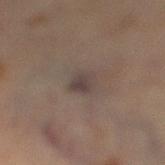Recorded during total-body skin imaging; not selected for excision or biopsy. Approximately 3 mm at its widest. A roughly 15 mm field-of-view crop from a total-body skin photograph. The lesion is on the right lower leg. A female patient roughly 70 years of age. Imaged with cross-polarized lighting. Automated image analysis of the tile measured a lesion area of about 5 mm², a shape eccentricity near 0.7, and a shape-asymmetry score of about 0.25 (0 = symmetric). The analysis additionally found a color-variation rating of about 3.5/10 and peripheral color asymmetry of about 1.